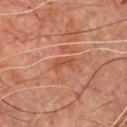<lesion>
<biopsy_status>not biopsied; imaged during a skin examination</biopsy_status>
<lighting>cross-polarized</lighting>
<site>chest</site>
<image>
  <source>total-body photography crop</source>
  <field_of_view_mm>15</field_of_view_mm>
</image>
<patient>
  <sex>male</sex>
  <age_approx>60</age_approx>
</patient>
</lesion>